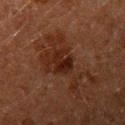No biopsy was performed on this lesion — it was imaged during a full skin examination and was not determined to be concerning. The subject is a male about 60 years old. Located on the left upper arm. This is a cross-polarized tile. A 15 mm close-up tile from a total-body photography series done for melanoma screening.